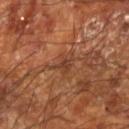Impression:
The lesion was tiled from a total-body skin photograph and was not biopsied.
Image and clinical context:
The lesion is located on the left forearm. Imaged with cross-polarized lighting. A male patient in their 70s. A 15 mm close-up extracted from a 3D total-body photography capture.